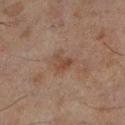notes: imaged on a skin check; not biopsied | lesion size: ≈2.5 mm | imaging modality: total-body-photography crop, ~15 mm field of view | patient: male, aged 43–47 | illumination: cross-polarized illumination | body site: the left lower leg.